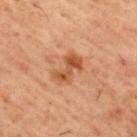No biopsy was performed on this lesion — it was imaged during a full skin examination and was not determined to be concerning.
From the upper back.
Approximately 4 mm at its widest.
This is a cross-polarized tile.
A male patient aged 53 to 57.
A close-up tile cropped from a whole-body skin photograph, about 15 mm across.
The total-body-photography lesion software estimated a footprint of about 7.5 mm², an eccentricity of roughly 0.8, and two-axis asymmetry of about 0.35.